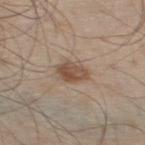{"lesion_size": {"long_diameter_mm_approx": 3.5}, "site": "left thigh", "lighting": "white-light", "patient": {"sex": "male", "age_approx": 60}, "image": {"source": "total-body photography crop", "field_of_view_mm": 15}}